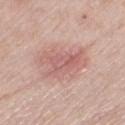Recorded during total-body skin imaging; not selected for excision or biopsy.
Imaged with white-light lighting.
A female subject aged 58–62.
The lesion's longest dimension is about 6 mm.
On the right thigh.
Cropped from a whole-body photographic skin survey; the tile spans about 15 mm.